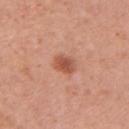| key | value |
|---|---|
| follow-up | no biopsy performed (imaged during a skin exam) |
| illumination | white-light illumination |
| body site | the left upper arm |
| patient | female, aged 33 to 37 |
| imaging modality | ~15 mm tile from a whole-body skin photo |
| automated lesion analysis | a lesion area of about 5 mm² and a symmetry-axis asymmetry near 0.2; a lesion color around L≈54 a*≈27 b*≈32 in CIELAB, roughly 11 lightness units darker than nearby skin, and a lesion-to-skin contrast of about 7.5 (normalized; higher = more distinct) |